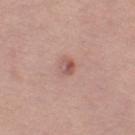Q: Was a biopsy performed?
A: imaged on a skin check; not biopsied
Q: Where on the body is the lesion?
A: the left thigh
Q: Illumination type?
A: white-light illumination
Q: Automated lesion metrics?
A: an area of roughly 3 mm², an eccentricity of roughly 0.35, and a shape-asymmetry score of about 0.25 (0 = symmetric)
Q: How large is the lesion?
A: about 2 mm
Q: Who is the patient?
A: female, about 40 years old
Q: How was this image acquired?
A: ~15 mm crop, total-body skin-cancer survey